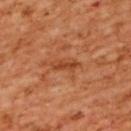Notes:
- imaging modality · ~15 mm tile from a whole-body skin photo
- lighting · cross-polarized
- patient · female, aged 53 to 57
- image-analysis metrics · a lesion color around L≈47 a*≈29 b*≈40 in CIELAB and a lesion–skin lightness drop of about 9; lesion-presence confidence of about 95/100
- anatomic site · the back